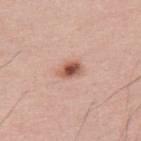follow-up: no biopsy performed (imaged during a skin exam) | automated metrics: a mean CIELAB color near L≈55 a*≈25 b*≈29, a lesion–skin lightness drop of about 16, and a normalized lesion–skin contrast near 10.5; a color-variation rating of about 6/10 and radial color variation of about 2; a nevus-likeness score of about 95/100 and lesion-presence confidence of about 100/100 | tile lighting: white-light illumination | imaging modality: ~15 mm crop, total-body skin-cancer survey | lesion diameter: ≈2.5 mm | patient: male, aged approximately 30 | site: the upper back.